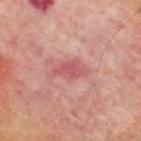  biopsy_status: not biopsied; imaged during a skin examination
  lesion_size:
    long_diameter_mm_approx: 4.0
  automated_metrics:
    area_mm2_approx: 6.5
    eccentricity: 0.85
    shape_asymmetry: 0.25
    border_irregularity_0_10: 2.5
    color_variation_0_10: 2.5
    peripheral_color_asymmetry: 0.5
  patient:
    sex: male
    age_approx: 70
  site: upper back
  image:
    source: total-body photography crop
    field_of_view_mm: 15